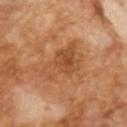workup: total-body-photography surveillance lesion; no biopsy | image source: ~15 mm crop, total-body skin-cancer survey | diameter: about 7 mm | patient: male, approximately 65 years of age.